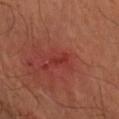<lesion>
<biopsy_status>not biopsied; imaged during a skin examination</biopsy_status>
<image>
  <source>total-body photography crop</source>
  <field_of_view_mm>15</field_of_view_mm>
</image>
<site>right forearm</site>
<lighting>cross-polarized</lighting>
<lesion_size>
  <long_diameter_mm_approx>3.0</long_diameter_mm_approx>
</lesion_size>
<patient>
  <sex>male</sex>
  <age_approx>55</age_approx>
</patient>
</lesion>